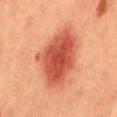The lesion was tiled from a total-body skin photograph and was not biopsied. A roughly 15 mm field-of-view crop from a total-body skin photograph. The total-body-photography lesion software estimated a shape-asymmetry score of about 0.2 (0 = symmetric). The software also gave an average lesion color of about L≈44 a*≈29 b*≈30 (CIELAB) and a normalized lesion–skin contrast near 9.5. The analysis additionally found a classifier nevus-likeness of about 100/100 and lesion-presence confidence of about 100/100. About 8 mm across. From the back. The tile uses cross-polarized illumination. A male subject, roughly 40 years of age.